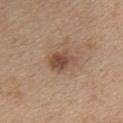{"biopsy_status": "not biopsied; imaged during a skin examination", "site": "chest", "patient": {"sex": "female", "age_approx": 45}, "image": {"source": "total-body photography crop", "field_of_view_mm": 15}, "automated_metrics": {"area_mm2_approx": 6.0, "eccentricity": 0.7, "shape_asymmetry": 0.3, "border_irregularity_0_10": 3.0, "color_variation_0_10": 2.5, "peripheral_color_asymmetry": 0.5, "nevus_likeness_0_100": 80, "lesion_detection_confidence_0_100": 100}, "lesion_size": {"long_diameter_mm_approx": 3.0}, "lighting": "white-light"}